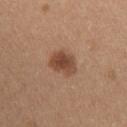Impression: No biopsy was performed on this lesion — it was imaged during a full skin examination and was not determined to be concerning. Clinical summary: Located on the head or neck. A region of skin cropped from a whole-body photographic capture, roughly 15 mm wide. Imaged with white-light lighting. Longest diameter approximately 3.5 mm. The subject is a female approximately 40 years of age.